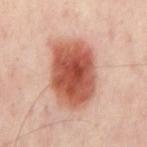Assessment: The lesion was tiled from a total-body skin photograph and was not biopsied. Image and clinical context: The lesion is located on the mid back. Cropped from a total-body skin-imaging series; the visible field is about 15 mm. A subject aged 53–57. The tile uses cross-polarized illumination.